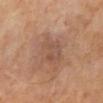Notes:
- biopsy status · total-body-photography surveillance lesion; no biopsy
- TBP lesion metrics · a lesion-to-skin contrast of about 4.5 (normalized; higher = more distinct); a border-irregularity rating of about 2.5/10 and radial color variation of about 1
- imaging modality · 15 mm crop, total-body photography
- patient · male, aged around 70
- lesion diameter · about 5 mm
- site · the mid back
- illumination · cross-polarized